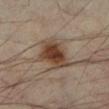Findings:
* notes · catalogued during a skin exam; not biopsied
* patient · male, in their mid- to late 50s
* anatomic site · the leg
* acquisition · ~15 mm crop, total-body skin-cancer survey
* diameter · about 5 mm
* lighting · cross-polarized